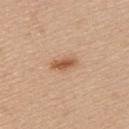follow-up: total-body-photography surveillance lesion; no biopsy | acquisition: ~15 mm tile from a whole-body skin photo | patient: female, approximately 45 years of age | site: the back.